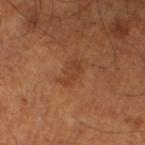The lesion was photographed on a routine skin check and not biopsied; there is no pathology result. The lesion's longest dimension is about 3 mm. On the leg. A roughly 15 mm field-of-view crop from a total-body skin photograph. An algorithmic analysis of the crop reported a border-irregularity index near 4/10 and peripheral color asymmetry of about 0.5. A male subject, approximately 65 years of age.